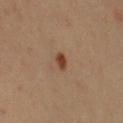* notes: imaged on a skin check; not biopsied
* subject: female, approximately 40 years of age
* site: the right upper arm
* diameter: about 2 mm
* imaging modality: ~15 mm crop, total-body skin-cancer survey